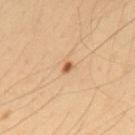This lesion was catalogued during total-body skin photography and was not selected for biopsy.
A male subject, aged around 35.
The lesion is located on the upper back.
An algorithmic analysis of the crop reported an area of roughly 1.5 mm², a shape eccentricity near 0.7, and a shape-asymmetry score of about 0.3 (0 = symmetric). It also reported an average lesion color of about L≈57 a*≈23 b*≈37 (CIELAB), about 14 CIELAB-L* units darker than the surrounding skin, and a normalized lesion–skin contrast near 9. It also reported a border-irregularity index near 2.5/10, a color-variation rating of about 0/10, and radial color variation of about 0. And it measured an automated nevus-likeness rating near 80 out of 100 and lesion-presence confidence of about 100/100.
A region of skin cropped from a whole-body photographic capture, roughly 15 mm wide.
The recorded lesion diameter is about 1.5 mm.
The tile uses cross-polarized illumination.